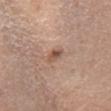The lesion was photographed on a routine skin check and not biopsied; there is no pathology result.